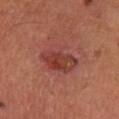| key | value |
|---|---|
| biopsy status | catalogued during a skin exam; not biopsied |
| tile lighting | cross-polarized |
| size | about 5 mm |
| acquisition | total-body-photography crop, ~15 mm field of view |
| automated metrics | an area of roughly 12 mm², an outline eccentricity of about 0.75 (0 = round, 1 = elongated), and a shape-asymmetry score of about 0.25 (0 = symmetric) |
| body site | the left lower leg |
| patient | male, about 55 years old |